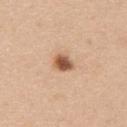The lesion was tiled from a total-body skin photograph and was not biopsied. Automated tile analysis of the lesion measured internal color variation of about 3.5 on a 0–10 scale and peripheral color asymmetry of about 1. Located on the upper back. About 2.5 mm across. The patient is a female roughly 55 years of age. This is a white-light tile. A 15 mm close-up extracted from a 3D total-body photography capture.